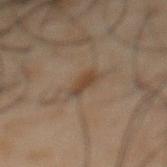follow-up: imaged on a skin check; not biopsied | site: the front of the torso | automated metrics: an area of roughly 3 mm²; a mean CIELAB color near L≈31 a*≈12 b*≈22, about 7 CIELAB-L* units darker than the surrounding skin, and a lesion-to-skin contrast of about 7 (normalized; higher = more distinct); border irregularity of about 3.5 on a 0–10 scale and a peripheral color-asymmetry measure near 0; a nevus-likeness score of about 55/100 and lesion-presence confidence of about 100/100 | diameter: ~2.5 mm (longest diameter) | lighting: cross-polarized illumination | patient: male, in their 50s | image source: 15 mm crop, total-body photography.